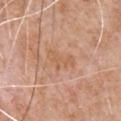Part of a total-body skin-imaging series; this lesion was reviewed on a skin check and was not flagged for biopsy.
The lesion is located on the chest.
A 15 mm crop from a total-body photograph taken for skin-cancer surveillance.
The lesion's longest dimension is about 3.5 mm.
The subject is a male in their mid- to late 60s.
The lesion-visualizer software estimated a lesion area of about 6 mm² and an eccentricity of roughly 0.8. The software also gave a lesion color around L≈60 a*≈22 b*≈34 in CIELAB, a lesion–skin lightness drop of about 5, and a lesion-to-skin contrast of about 4.5 (normalized; higher = more distinct). And it measured an automated nevus-likeness rating near 0 out of 100 and a detector confidence of about 100 out of 100 that the crop contains a lesion.
This is a white-light tile.